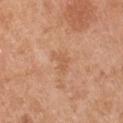No biopsy was performed on this lesion — it was imaged during a full skin examination and was not determined to be concerning.
The tile uses white-light illumination.
A region of skin cropped from a whole-body photographic capture, roughly 15 mm wide.
Automated image analysis of the tile measured a shape eccentricity near 0.8 and a symmetry-axis asymmetry near 0.6. The analysis additionally found a normalized border contrast of about 4.5.
On the left upper arm.
The recorded lesion diameter is about 3 mm.
A male subject, about 55 years old.